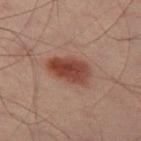Recorded during total-body skin imaging; not selected for excision or biopsy. A male subject, in their 40s. Longest diameter approximately 4.5 mm. On the left thigh. A roughly 15 mm field-of-view crop from a total-body skin photograph. The lesion-visualizer software estimated a lesion area of about 11 mm², a shape eccentricity near 0.8, and a symmetry-axis asymmetry near 0.15. And it measured an average lesion color of about L≈33 a*≈20 b*≈22 (CIELAB), roughly 10 lightness units darker than nearby skin, and a normalized border contrast of about 10. And it measured border irregularity of about 2 on a 0–10 scale and a peripheral color-asymmetry measure near 1.5.